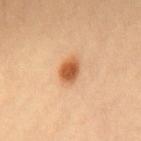Q: Was this lesion biopsied?
A: total-body-photography surveillance lesion; no biopsy
Q: What is the anatomic site?
A: the upper back
Q: How was this image acquired?
A: total-body-photography crop, ~15 mm field of view
Q: Automated lesion metrics?
A: a lesion area of about 5.5 mm² and a symmetry-axis asymmetry near 0.2; a detector confidence of about 100 out of 100 that the crop contains a lesion
Q: What is the lesion's diameter?
A: about 3 mm
Q: What are the patient's age and sex?
A: female, aged around 35